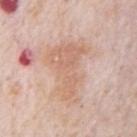biopsy status = no biopsy performed (imaged during a skin exam) | location = the chest | image = ~15 mm tile from a whole-body skin photo | automated lesion analysis = a mean CIELAB color near L≈66 a*≈19 b*≈29 and about 7 CIELAB-L* units darker than the surrounding skin; a within-lesion color-variation index near 3/10 and a peripheral color-asymmetry measure near 1; an automated nevus-likeness rating near 0 out of 100 and lesion-presence confidence of about 90/100 | tile lighting = white-light illumination | subject = male, aged 78–82 | lesion size = about 7.5 mm.